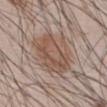• biopsy status: imaged on a skin check; not biopsied
• tile lighting: white-light illumination
• size: about 6 mm
• patient: male, in their mid- to late 50s
• anatomic site: the abdomen
• acquisition: total-body-photography crop, ~15 mm field of view